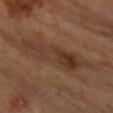The lesion was photographed on a routine skin check and not biopsied; there is no pathology result. The tile uses cross-polarized illumination. A female patient aged around 60. A region of skin cropped from a whole-body photographic capture, roughly 15 mm wide. The lesion is on the arm. Measured at roughly 9.5 mm in maximum diameter.